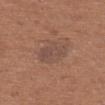Assessment:
Captured during whole-body skin photography for melanoma surveillance; the lesion was not biopsied.
Acquisition and patient details:
The lesion is located on the upper back. The patient is a female in their mid-60s. Approximately 4 mm at its widest. The total-body-photography lesion software estimated a footprint of about 9.5 mm², an outline eccentricity of about 0.5 (0 = round, 1 = elongated), and two-axis asymmetry of about 0.2. The analysis additionally found border irregularity of about 2 on a 0–10 scale, internal color variation of about 2.5 on a 0–10 scale, and a peripheral color-asymmetry measure near 1. A region of skin cropped from a whole-body photographic capture, roughly 15 mm wide. Captured under white-light illumination.